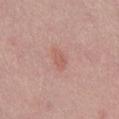Imaged during a routine full-body skin examination; the lesion was not biopsied and no histopathology is available.
The lesion is on the right thigh.
Cropped from a total-body skin-imaging series; the visible field is about 15 mm.
A male subject aged around 40.